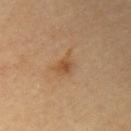Clinical impression:
Part of a total-body skin-imaging series; this lesion was reviewed on a skin check and was not flagged for biopsy.
Clinical summary:
Cropped from a total-body skin-imaging series; the visible field is about 15 mm. Longest diameter approximately 2.5 mm. The tile uses cross-polarized illumination. The lesion is located on the right upper arm. The patient is a male in their mid-80s or older.